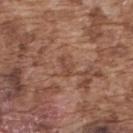Recorded during total-body skin imaging; not selected for excision or biopsy. Located on the upper back. Cropped from a total-body skin-imaging series; the visible field is about 15 mm. A male patient aged approximately 75.